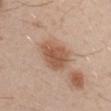Impression: The lesion was photographed on a routine skin check and not biopsied; there is no pathology result. Clinical summary: From the right upper arm. A male patient aged 28 to 32. A 15 mm close-up tile from a total-body photography series done for melanoma screening. Longest diameter approximately 5 mm.